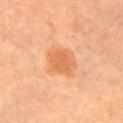The lesion was photographed on a routine skin check and not biopsied; there is no pathology result.
The recorded lesion diameter is about 4 mm.
An algorithmic analysis of the crop reported a lesion–skin lightness drop of about 9 and a normalized lesion–skin contrast near 6.5. It also reported internal color variation of about 3 on a 0–10 scale and peripheral color asymmetry of about 1. And it measured a nevus-likeness score of about 95/100 and a lesion-detection confidence of about 100/100.
Located on the left upper arm.
A female subject aged around 65.
Captured under cross-polarized illumination.
Cropped from a whole-body photographic skin survey; the tile spans about 15 mm.